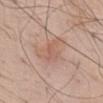{"biopsy_status": "not biopsied; imaged during a skin examination", "site": "chest", "image": {"source": "total-body photography crop", "field_of_view_mm": 15}, "patient": {"sex": "male", "age_approx": 60}}